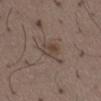Imaged with white-light lighting.
A male subject, approximately 50 years of age.
On the right thigh.
A roughly 15 mm field-of-view crop from a total-body skin photograph.